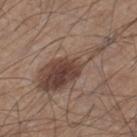follow-up: catalogued during a skin exam; not biopsied | automated lesion analysis: a lesion area of about 21 mm², an eccentricity of roughly 0.95, and a shape-asymmetry score of about 0.55 (0 = symmetric); a lesion color around L≈43 a*≈16 b*≈23 in CIELAB, a lesion–skin lightness drop of about 11, and a lesion-to-skin contrast of about 8.5 (normalized; higher = more distinct); border irregularity of about 8 on a 0–10 scale, internal color variation of about 6.5 on a 0–10 scale, and radial color variation of about 2.5 | imaging modality: ~15 mm crop, total-body skin-cancer survey | illumination: white-light | site: the right lower leg | patient: male, in their mid- to late 40s | size: about 10 mm.